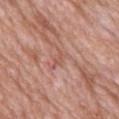notes=catalogued during a skin exam; not biopsied | lighting=white-light | imaging modality=total-body-photography crop, ~15 mm field of view | site=the upper back | automated metrics=a footprint of about 3.5 mm²; a lesion color around L≈56 a*≈24 b*≈27 in CIELAB, roughly 7 lightness units darker than nearby skin, and a normalized lesion–skin contrast near 4.5 | patient=female, aged 68 to 72 | lesion size=about 3 mm.